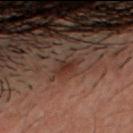This lesion was catalogued during total-body skin photography and was not selected for biopsy.
The lesion is located on the head or neck.
A male subject, aged around 30.
A 15 mm crop from a total-body photograph taken for skin-cancer surveillance.